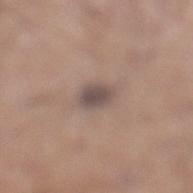The lesion was photographed on a routine skin check and not biopsied; there is no pathology result.